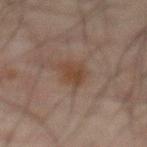The lesion was tiled from a total-body skin photograph and was not biopsied. A male subject aged 68–72. Located on the front of the torso. Automated image analysis of the tile measured an area of roughly 5.5 mm², an outline eccentricity of about 0.75 (0 = round, 1 = elongated), and two-axis asymmetry of about 0.3. It also reported an average lesion color of about L≈34 a*≈14 b*≈23 (CIELAB), a lesion–skin lightness drop of about 6, and a normalized lesion–skin contrast near 7. And it measured a border-irregularity index near 3/10 and a within-lesion color-variation index near 2/10. A roughly 15 mm field-of-view crop from a total-body skin photograph.